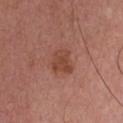workup = catalogued during a skin exam; not biopsied.